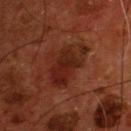The lesion was photographed on a routine skin check and not biopsied; there is no pathology result. Cropped from a total-body skin-imaging series; the visible field is about 15 mm. On the chest. An algorithmic analysis of the crop reported a footprint of about 15 mm², a shape eccentricity near 0.8, and a shape-asymmetry score of about 0.4 (0 = symmetric). It also reported a border-irregularity rating of about 5.5/10, a within-lesion color-variation index near 4/10, and radial color variation of about 1.5. And it measured a nevus-likeness score of about 15/100 and lesion-presence confidence of about 100/100. The subject is a male aged approximately 55. Longest diameter approximately 6 mm. The tile uses cross-polarized illumination.